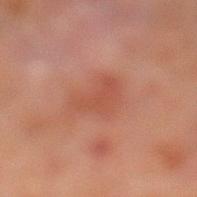No biopsy was performed on this lesion — it was imaged during a full skin examination and was not determined to be concerning. A male patient, aged 68–72. On the left lower leg. A lesion tile, about 15 mm wide, cut from a 3D total-body photograph.